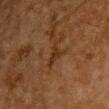The lesion was photographed on a routine skin check and not biopsied; there is no pathology result. A male subject aged approximately 60. The total-body-photography lesion software estimated a footprint of about 3 mm², an outline eccentricity of about 0.7 (0 = round, 1 = elongated), and two-axis asymmetry of about 0.5. The recorded lesion diameter is about 2.5 mm. From the chest. Cropped from a whole-body photographic skin survey; the tile spans about 15 mm.